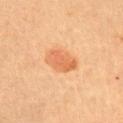workup: imaged on a skin check; not biopsied | patient: female, aged 58 to 62 | automated lesion analysis: an eccentricity of roughly 0.75 and two-axis asymmetry of about 0.2; border irregularity of about 2 on a 0–10 scale and a color-variation rating of about 3/10; a classifier nevus-likeness of about 90/100 and a lesion-detection confidence of about 100/100 | lesion size: ~4 mm (longest diameter) | acquisition: 15 mm crop, total-body photography | location: the left upper arm.